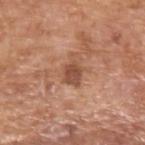No biopsy was performed on this lesion — it was imaged during a full skin examination and was not determined to be concerning. The lesion is on the arm. About 3.5 mm across. Cropped from a whole-body photographic skin survey; the tile spans about 15 mm. A male subject, aged around 75. Automated tile analysis of the lesion measured a lesion area of about 6 mm² and an eccentricity of roughly 0.8. The analysis additionally found radial color variation of about 1. The software also gave a nevus-likeness score of about 0/100 and lesion-presence confidence of about 100/100.